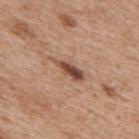{"lighting": "white-light", "site": "mid back", "lesion_size": {"long_diameter_mm_approx": 4.5}, "automated_metrics": {"area_mm2_approx": 5.5, "eccentricity": 0.95, "shape_asymmetry": 0.35, "nevus_likeness_0_100": 0}, "image": {"source": "total-body photography crop", "field_of_view_mm": 15}, "patient": {"sex": "male", "age_approx": 65}}